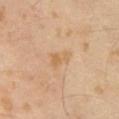Impression: Imaged during a routine full-body skin examination; the lesion was not biopsied and no histopathology is available. Acquisition and patient details: Measured at roughly 3 mm in maximum diameter. Automated tile analysis of the lesion measured a detector confidence of about 100 out of 100 that the crop contains a lesion. Cropped from a total-body skin-imaging series; the visible field is about 15 mm. Imaged with cross-polarized lighting. The subject is a male approximately 65 years of age.